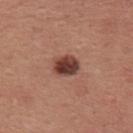Captured during whole-body skin photography for melanoma surveillance; the lesion was not biopsied.
A 15 mm close-up extracted from a 3D total-body photography capture.
Located on the back.
About 3 mm across.
Automated image analysis of the tile measured a normalized border contrast of about 11.5. The software also gave a border-irregularity index near 1/10, internal color variation of about 5 on a 0–10 scale, and a peripheral color-asymmetry measure near 1.5.
A male patient, aged approximately 40.
The tile uses white-light illumination.